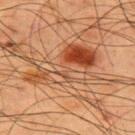workup: catalogued during a skin exam; not biopsied | automated metrics: a mean CIELAB color near L≈40 a*≈20 b*≈30, a lesion–skin lightness drop of about 8, and a normalized border contrast of about 7; border irregularity of about 8.5 on a 0–10 scale, a color-variation rating of about 10/10, and peripheral color asymmetry of about 3.5; an automated nevus-likeness rating near 30 out of 100 | anatomic site: the upper back | subject: male, aged 58 to 62 | imaging modality: ~15 mm tile from a whole-body skin photo | lesion diameter: ~10 mm (longest diameter) | tile lighting: cross-polarized illumination.